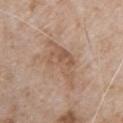Q: Was a biopsy performed?
A: catalogued during a skin exam; not biopsied
Q: Where on the body is the lesion?
A: the chest
Q: How was this image acquired?
A: ~15 mm tile from a whole-body skin photo
Q: Who is the patient?
A: male, aged approximately 80